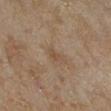The lesion was photographed on a routine skin check and not biopsied; there is no pathology result. This is a cross-polarized tile. A female subject, roughly 60 years of age. On the right lower leg. Longest diameter approximately 2.5 mm. A 15 mm crop from a total-body photograph taken for skin-cancer surveillance.